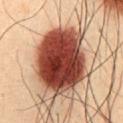Impression:
Recorded during total-body skin imaging; not selected for excision or biopsy.
Background:
A 15 mm close-up tile from a total-body photography series done for melanoma screening. The lesion is on the chest. The tile uses cross-polarized illumination. About 8.5 mm across. The patient is a male in their mid- to late 30s.